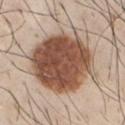biopsy status: catalogued during a skin exam; not biopsied | lighting: white-light illumination | lesion diameter: ~8 mm (longest diameter) | image: 15 mm crop, total-body photography | site: the chest | patient: male, roughly 30 years of age.